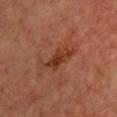This lesion was catalogued during total-body skin photography and was not selected for biopsy. A region of skin cropped from a whole-body photographic capture, roughly 15 mm wide. The lesion is located on the chest. The subject is a female about 40 years old. About 4.5 mm across.